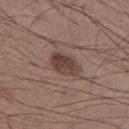Q: What is the anatomic site?
A: the left thigh
Q: What are the patient's age and sex?
A: male, aged 33–37
Q: How was this image acquired?
A: total-body-photography crop, ~15 mm field of view